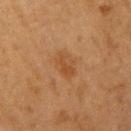Recorded during total-body skin imaging; not selected for excision or biopsy.
Captured under cross-polarized illumination.
The lesion's longest dimension is about 3 mm.
The lesion-visualizer software estimated an area of roughly 6 mm², an outline eccentricity of about 0.65 (0 = round, 1 = elongated), and two-axis asymmetry of about 0.15. The analysis additionally found a mean CIELAB color near L≈39 a*≈19 b*≈33, roughly 6 lightness units darker than nearby skin, and a lesion-to-skin contrast of about 5.5 (normalized; higher = more distinct). The software also gave a within-lesion color-variation index near 2.5/10 and a peripheral color-asymmetry measure near 1. And it measured a lesion-detection confidence of about 100/100.
A 15 mm close-up extracted from a 3D total-body photography capture.
From the arm.
A female subject, aged approximately 55.